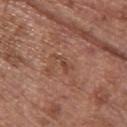Assessment: Captured during whole-body skin photography for melanoma surveillance; the lesion was not biopsied. Image and clinical context: Measured at roughly 2.5 mm in maximum diameter. The subject is a female in their mid- to late 60s. This image is a 15 mm lesion crop taken from a total-body photograph. From the upper back. Captured under white-light illumination.